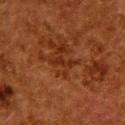Part of a total-body skin-imaging series; this lesion was reviewed on a skin check and was not flagged for biopsy.
A 15 mm crop from a total-body photograph taken for skin-cancer surveillance.
Captured under cross-polarized illumination.
About 2.5 mm across.
From the back.
A female patient, in their 50s.
Automated image analysis of the tile measured an area of roughly 2.5 mm², an outline eccentricity of about 0.9 (0 = round, 1 = elongated), and a symmetry-axis asymmetry near 0.45. It also reported border irregularity of about 5.5 on a 0–10 scale, a within-lesion color-variation index near 0/10, and radial color variation of about 0.